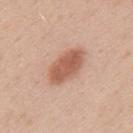follow-up=total-body-photography surveillance lesion; no biopsy
image=~15 mm crop, total-body skin-cancer survey
size=~5.5 mm (longest diameter)
illumination=white-light
TBP lesion metrics=an area of roughly 12 mm² and two-axis asymmetry of about 0.1
subject=male, aged approximately 35
anatomic site=the upper back